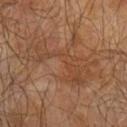Q: Was a biopsy performed?
A: imaged on a skin check; not biopsied
Q: What did automated image analysis measure?
A: a border-irregularity rating of about 10/10, a within-lesion color-variation index near 3/10, and radial color variation of about 1; an automated nevus-likeness rating near 0 out of 100 and a detector confidence of about 75 out of 100 that the crop contains a lesion
Q: What kind of image is this?
A: ~15 mm tile from a whole-body skin photo
Q: Where on the body is the lesion?
A: the left upper arm
Q: How large is the lesion?
A: ≈9 mm
Q: What lighting was used for the tile?
A: cross-polarized
Q: Who is the patient?
A: male, aged around 65